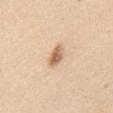follow-up: no biopsy performed (imaged during a skin exam); anatomic site: the chest; lighting: white-light illumination; subject: male, aged 43–47; size: ≈3 mm; image source: ~15 mm tile from a whole-body skin photo.